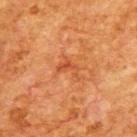The lesion was photographed on a routine skin check and not biopsied; there is no pathology result.
From the upper back.
A 15 mm close-up tile from a total-body photography series done for melanoma screening.
Measured at roughly 3 mm in maximum diameter.
A male patient approximately 80 years of age.